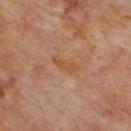Clinical impression:
Part of a total-body skin-imaging series; this lesion was reviewed on a skin check and was not flagged for biopsy.
Background:
A 15 mm crop from a total-body photograph taken for skin-cancer surveillance. Measured at roughly 3.5 mm in maximum diameter. From the back. A male subject, roughly 70 years of age.